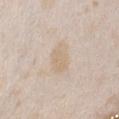Imaged during a routine full-body skin examination; the lesion was not biopsied and no histopathology is available. A close-up tile cropped from a whole-body skin photograph, about 15 mm across. About 4 mm across. A female patient aged around 25. Automated tile analysis of the lesion measured a lesion area of about 7.5 mm², an eccentricity of roughly 0.8, and a symmetry-axis asymmetry near 0.15. And it measured a mean CIELAB color near L≈69 a*≈11 b*≈30, a lesion–skin lightness drop of about 6, and a lesion-to-skin contrast of about 5 (normalized; higher = more distinct). And it measured border irregularity of about 1.5 on a 0–10 scale, a within-lesion color-variation index near 1.5/10, and a peripheral color-asymmetry measure near 0.5. Captured under white-light illumination. The lesion is located on the chest.